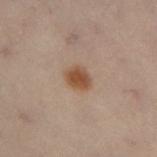Clinical impression:
The lesion was photographed on a routine skin check and not biopsied; there is no pathology result.
Background:
On the leg. A lesion tile, about 15 mm wide, cut from a 3D total-body photograph. Captured under cross-polarized illumination. The patient is a female aged 48 to 52.